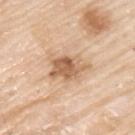Q: Is there a histopathology result?
A: imaged on a skin check; not biopsied
Q: What kind of image is this?
A: total-body-photography crop, ~15 mm field of view
Q: Lesion size?
A: about 5 mm
Q: Patient demographics?
A: male, aged approximately 80
Q: Where on the body is the lesion?
A: the upper back